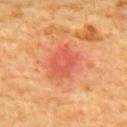Captured during whole-body skin photography for melanoma surveillance; the lesion was not biopsied. Longest diameter approximately 4 mm. A close-up tile cropped from a whole-body skin photograph, about 15 mm across. The patient is a male aged around 60. Automated tile analysis of the lesion measured an area of roughly 8.5 mm², an eccentricity of roughly 0.7, and a shape-asymmetry score of about 0.25 (0 = symmetric). And it measured a lesion–skin lightness drop of about 8 and a normalized border contrast of about 5.5. Located on the upper back. The tile uses cross-polarized illumination.